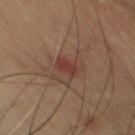- notes — no biopsy performed (imaged during a skin exam)
- body site — the chest
- patient — male, about 70 years old
- imaging modality — 15 mm crop, total-body photography
- tile lighting — cross-polarized illumination
- diameter — ≈3 mm